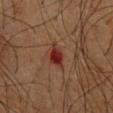| feature | finding |
|---|---|
| notes | total-body-photography surveillance lesion; no biopsy |
| illumination | cross-polarized |
| image | ~15 mm tile from a whole-body skin photo |
| patient | male, aged approximately 65 |
| body site | the chest |